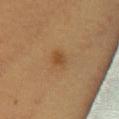| field | value |
|---|---|
| workup | imaged on a skin check; not biopsied |
| lighting | cross-polarized illumination |
| lesion size | ~2.5 mm (longest diameter) |
| acquisition | total-body-photography crop, ~15 mm field of view |
| patient | female, roughly 60 years of age |
| anatomic site | the chest |